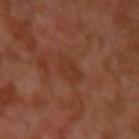Clinical impression:
Captured during whole-body skin photography for melanoma surveillance; the lesion was not biopsied.
Context:
The lesion is located on the left upper arm. A 15 mm close-up extracted from a 3D total-body photography capture. A male patient approximately 30 years of age.